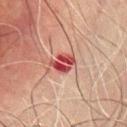An algorithmic analysis of the crop reported a shape eccentricity near 0.55 and two-axis asymmetry of about 0.25. The analysis additionally found a mean CIELAB color near L≈40 a*≈32 b*≈23, about 13 CIELAB-L* units darker than the surrounding skin, and a normalized lesion–skin contrast near 10.5. Cropped from a total-body skin-imaging series; the visible field is about 15 mm. The lesion is located on the mid back. The tile uses cross-polarized illumination. A male patient aged 68–72.